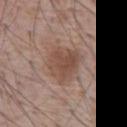<lesion>
  <biopsy_status>not biopsied; imaged during a skin examination</biopsy_status>
  <site>abdomen</site>
  <patient>
    <sex>male</sex>
    <age_approx>70</age_approx>
  </patient>
  <automated_metrics>
    <area_mm2_approx>13.0</area_mm2_approx>
    <eccentricity>0.5</eccentricity>
    <shape_asymmetry>0.25</shape_asymmetry>
    <cielab_L>49</cielab_L>
    <cielab_a>18</cielab_a>
    <cielab_b>26</cielab_b>
    <nevus_likeness_0_100>55</nevus_likeness_0_100>
    <lesion_detection_confidence_0_100>100</lesion_detection_confidence_0_100>
  </automated_metrics>
  <lesion_size>
    <long_diameter_mm_approx>5.0</long_diameter_mm_approx>
  </lesion_size>
  <image>
    <source>total-body photography crop</source>
    <field_of_view_mm>15</field_of_view_mm>
  </image>
</lesion>